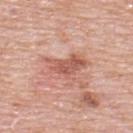Assessment:
The lesion was tiled from a total-body skin photograph and was not biopsied.
Acquisition and patient details:
A male patient, roughly 80 years of age. The tile uses white-light illumination. An algorithmic analysis of the crop reported a lesion area of about 10 mm² and an outline eccentricity of about 0.85 (0 = round, 1 = elongated). The analysis additionally found a border-irregularity rating of about 3.5/10, a color-variation rating of about 5/10, and a peripheral color-asymmetry measure near 2. It also reported a nevus-likeness score of about 0/100 and lesion-presence confidence of about 100/100. The lesion is on the upper back. Measured at roughly 5 mm in maximum diameter. Cropped from a whole-body photographic skin survey; the tile spans about 15 mm.